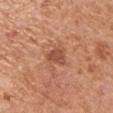Q: Was a biopsy performed?
A: imaged on a skin check; not biopsied
Q: What is the imaging modality?
A: ~15 mm crop, total-body skin-cancer survey
Q: How large is the lesion?
A: about 2.5 mm
Q: Automated lesion metrics?
A: a border-irregularity rating of about 3.5/10, a within-lesion color-variation index near 1/10, and radial color variation of about 0.5
Q: What are the patient's age and sex?
A: male, aged 63 to 67
Q: What is the anatomic site?
A: the left upper arm
Q: What lighting was used for the tile?
A: white-light